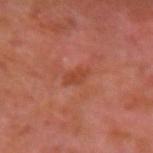<lesion>
  <biopsy_status>not biopsied; imaged during a skin examination</biopsy_status>
  <image>
    <source>total-body photography crop</source>
    <field_of_view_mm>15</field_of_view_mm>
  </image>
  <patient>
    <sex>male</sex>
    <age_approx>30</age_approx>
  </patient>
  <site>left arm</site>
</lesion>